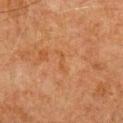notes=imaged on a skin check; not biopsied
automated lesion analysis=a mean CIELAB color near L≈41 a*≈21 b*≈33, a lesion–skin lightness drop of about 4, and a normalized lesion–skin contrast near 5; an automated nevus-likeness rating near 0 out of 100
location=the abdomen
image source=15 mm crop, total-body photography
lesion size=about 3 mm
illumination=cross-polarized illumination
patient=male, aged around 80